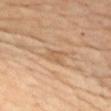| key | value |
|---|---|
| notes | imaged on a skin check; not biopsied |
| patient | female, aged 58 to 62 |
| imaging modality | total-body-photography crop, ~15 mm field of view |
| location | the front of the torso |